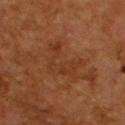{"patient": {"sex": "male", "age_approx": 55}, "image": {"source": "total-body photography crop", "field_of_view_mm": 15}, "lesion_size": {"long_diameter_mm_approx": 5.0}, "site": "upper back"}